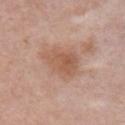Clinical impression:
Part of a total-body skin-imaging series; this lesion was reviewed on a skin check and was not flagged for biopsy.